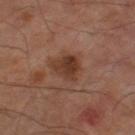Imaged during a routine full-body skin examination; the lesion was not biopsied and no histopathology is available.
A male patient aged 63–67.
The lesion's longest dimension is about 3 mm.
A roughly 15 mm field-of-view crop from a total-body skin photograph.
Located on the left thigh.